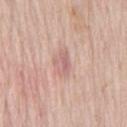  biopsy_status: not biopsied; imaged during a skin examination
  image:
    source: total-body photography crop
    field_of_view_mm: 15
  site: lower back
  automated_metrics:
    area_mm2_approx: 4.5
    eccentricity: 0.8
    shape_asymmetry: 0.35
    border_irregularity_0_10: 3.5
    color_variation_0_10: 3.0
    peripheral_color_asymmetry: 1.0
    nevus_likeness_0_100: 0
  lighting: white-light
  lesion_size:
    long_diameter_mm_approx: 3.5
  patient:
    sex: male
    age_approx: 70